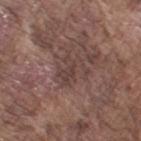<lesion>
  <biopsy_status>not biopsied; imaged during a skin examination</biopsy_status>
  <patient>
    <sex>male</sex>
    <age_approx>75</age_approx>
  </patient>
  <image>
    <source>total-body photography crop</source>
    <field_of_view_mm>15</field_of_view_mm>
  </image>
  <lesion_size>
    <long_diameter_mm_approx>4.0</long_diameter_mm_approx>
  </lesion_size>
  <site>arm</site>
  <automated_metrics>
    <eccentricity>0.5</eccentricity>
    <shape_asymmetry>0.45</shape_asymmetry>
    <vs_skin_contrast_norm>5.5</vs_skin_contrast_norm>
    <border_irregularity_0_10>8.5</border_irregularity_0_10>
    <color_variation_0_10>2.0</color_variation_0_10>
    <peripheral_color_asymmetry>0.5</peripheral_color_asymmetry>
  </automated_metrics>
  <lighting>white-light</lighting>
</lesion>